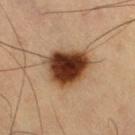{
  "biopsy_status": "not biopsied; imaged during a skin examination",
  "lesion_size": {
    "long_diameter_mm_approx": 5.5
  },
  "image": {
    "source": "total-body photography crop",
    "field_of_view_mm": 15
  },
  "site": "right thigh",
  "patient": {
    "sex": "male",
    "age_approx": 60
  },
  "lighting": "cross-polarized"
}